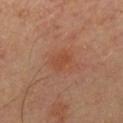<case>
<biopsy_status>not biopsied; imaged during a skin examination</biopsy_status>
<automated_metrics>
  <area_mm2_approx>3.0</area_mm2_approx>
  <shape_asymmetry>0.25</shape_asymmetry>
  <nevus_likeness_0_100>10</nevus_likeness_0_100>
  <lesion_detection_confidence_0_100>100</lesion_detection_confidence_0_100>
</automated_metrics>
<patient>
  <sex>male</sex>
  <age_approx>55</age_approx>
</patient>
<site>chest</site>
<lighting>cross-polarized</lighting>
<image>
  <source>total-body photography crop</source>
  <field_of_view_mm>15</field_of_view_mm>
</image>
<lesion_size>
  <long_diameter_mm_approx>2.5</long_diameter_mm_approx>
</lesion_size>
</case>